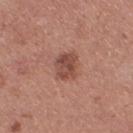Part of a total-body skin-imaging series; this lesion was reviewed on a skin check and was not flagged for biopsy. Automated image analysis of the tile measured an area of roughly 7 mm² and a symmetry-axis asymmetry near 0.2. And it measured a border-irregularity rating of about 2/10, a color-variation rating of about 4/10, and peripheral color asymmetry of about 1.5. The analysis additionally found a nevus-likeness score of about 60/100 and lesion-presence confidence of about 100/100. This is a white-light tile. The lesion is located on the right thigh. Cropped from a total-body skin-imaging series; the visible field is about 15 mm. A female patient, approximately 30 years of age.